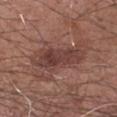Part of a total-body skin-imaging series; this lesion was reviewed on a skin check and was not flagged for biopsy. A male subject in their mid-70s. A 15 mm close-up extracted from a 3D total-body photography capture. The lesion is on the arm. The tile uses white-light illumination. Longest diameter approximately 6 mm.